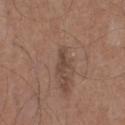{"lighting": "white-light", "patient": {"sex": "male", "age_approx": 75}, "image": {"source": "total-body photography crop", "field_of_view_mm": 15}, "site": "leg"}